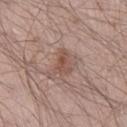Q: How large is the lesion?
A: ~3 mm (longest diameter)
Q: Lesion location?
A: the right lower leg
Q: What did automated image analysis measure?
A: an average lesion color of about L≈51 a*≈19 b*≈26 (CIELAB), about 8 CIELAB-L* units darker than the surrounding skin, and a lesion-to-skin contrast of about 6.5 (normalized; higher = more distinct); a nevus-likeness score of about 25/100 and a detector confidence of about 100 out of 100 that the crop contains a lesion
Q: What kind of image is this?
A: ~15 mm tile from a whole-body skin photo
Q: What are the patient's age and sex?
A: male, aged around 60